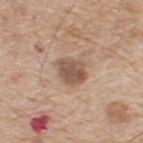{"biopsy_status": "not biopsied; imaged during a skin examination", "site": "upper back", "patient": {"sex": "male", "age_approx": 70}, "lighting": "white-light", "image": {"source": "total-body photography crop", "field_of_view_mm": 15}, "lesion_size": {"long_diameter_mm_approx": 3.5}, "automated_metrics": {"eccentricity": 0.5, "shape_asymmetry": 0.25, "cielab_L": 53, "cielab_a": 17, "cielab_b": 27, "vs_skin_darker_L": 12.0, "vs_skin_contrast_norm": 8.5, "nevus_likeness_0_100": 20, "lesion_detection_confidence_0_100": 100}}